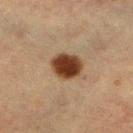workup: no biopsy performed (imaged during a skin exam) | lighting: cross-polarized | image-analysis metrics: a footprint of about 9 mm², an outline eccentricity of about 0.6 (0 = round, 1 = elongated), and a symmetry-axis asymmetry near 0.1; a mean CIELAB color near L≈31 a*≈18 b*≈27, about 16 CIELAB-L* units darker than the surrounding skin, and a normalized lesion–skin contrast near 14.5; a border-irregularity index near 1/10, a color-variation rating of about 3.5/10, and peripheral color asymmetry of about 1 | subject: female, roughly 55 years of age | image: ~15 mm tile from a whole-body skin photo | site: the left lower leg.